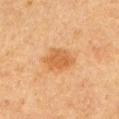Notes:
* follow-up: no biopsy performed (imaged during a skin exam)
* size: ~4 mm (longest diameter)
* tile lighting: cross-polarized illumination
* acquisition: ~15 mm crop, total-body skin-cancer survey
* patient: male, aged 63 to 67
* location: the left upper arm